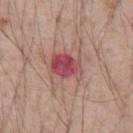Notes:
* biopsy status · catalogued during a skin exam; not biopsied
* tile lighting · white-light
* image source · total-body-photography crop, ~15 mm field of view
* patient · male, roughly 55 years of age
* body site · the abdomen
* lesion size · ≈3.5 mm